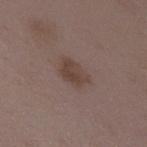The lesion was tiled from a total-body skin photograph and was not biopsied.
The lesion is located on the back.
A female subject in their mid- to late 30s.
A 15 mm crop from a total-body photograph taken for skin-cancer surveillance.